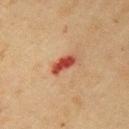workup: imaged on a skin check; not biopsied
subject: female, approximately 55 years of age
anatomic site: the arm
acquisition: ~15 mm crop, total-body skin-cancer survey
illumination: cross-polarized illumination
size: ≈3.5 mm
image-analysis metrics: a mean CIELAB color near L≈44 a*≈30 b*≈31, roughly 14 lightness units darker than nearby skin, and a lesion-to-skin contrast of about 10.5 (normalized; higher = more distinct); a border-irregularity rating of about 3/10 and a color-variation rating of about 3.5/10